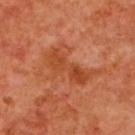Assessment: The lesion was photographed on a routine skin check and not biopsied; there is no pathology result. Acquisition and patient details: Longest diameter approximately 5.5 mm. Cropped from a whole-body photographic skin survey; the tile spans about 15 mm. The patient is a female about 40 years old. The lesion-visualizer software estimated an area of roughly 9.5 mm², a shape eccentricity near 0.95, and two-axis asymmetry of about 0.3. It also reported an average lesion color of about L≈47 a*≈32 b*≈40 (CIELAB) and a normalized lesion–skin contrast near 7. The analysis additionally found a classifier nevus-likeness of about 0/100 and lesion-presence confidence of about 100/100. The lesion is located on the upper back.